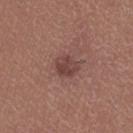Clinical impression:
Part of a total-body skin-imaging series; this lesion was reviewed on a skin check and was not flagged for biopsy.
Acquisition and patient details:
A 15 mm crop from a total-body photograph taken for skin-cancer surveillance. An algorithmic analysis of the crop reported an area of roughly 6 mm² and an eccentricity of roughly 0.5. It also reported a lesion color around L≈42 a*≈21 b*≈22 in CIELAB and a lesion–skin lightness drop of about 9. The analysis additionally found a border-irregularity rating of about 2/10, a color-variation rating of about 3/10, and radial color variation of about 1. And it measured a classifier nevus-likeness of about 30/100 and a lesion-detection confidence of about 100/100. A female patient, aged approximately 40. From the left thigh. This is a white-light tile. The recorded lesion diameter is about 3 mm.